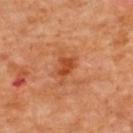Part of a total-body skin-imaging series; this lesion was reviewed on a skin check and was not flagged for biopsy.
The lesion's longest dimension is about 3 mm.
The lesion is on the upper back.
This is a cross-polarized tile.
A 15 mm close-up tile from a total-body photography series done for melanoma screening.
Automated image analysis of the tile measured a border-irregularity rating of about 3/10, internal color variation of about 1.5 on a 0–10 scale, and peripheral color asymmetry of about 0.5. The software also gave an automated nevus-likeness rating near 30 out of 100 and a detector confidence of about 100 out of 100 that the crop contains a lesion.
A female subject, aged approximately 50.